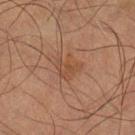{
  "biopsy_status": "not biopsied; imaged during a skin examination"
}